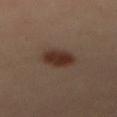Imaged during a routine full-body skin examination; the lesion was not biopsied and no histopathology is available. The subject is a male approximately 40 years of age. A 15 mm close-up extracted from a 3D total-body photography capture. The lesion-visualizer software estimated a within-lesion color-variation index near 2.5/10. And it measured a nevus-likeness score of about 100/100. Located on the mid back. The lesion's longest dimension is about 4.5 mm. Imaged with cross-polarized lighting.